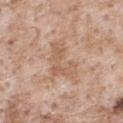The lesion was photographed on a routine skin check and not biopsied; there is no pathology result.
This is a white-light tile.
A lesion tile, about 15 mm wide, cut from a 3D total-body photograph.
The recorded lesion diameter is about 4.5 mm.
The patient is a male approximately 65 years of age.
Located on the chest.